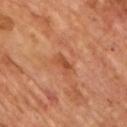Clinical impression:
Part of a total-body skin-imaging series; this lesion was reviewed on a skin check and was not flagged for biopsy.
Clinical summary:
A 15 mm close-up extracted from a 3D total-body photography capture. The patient is a male roughly 65 years of age. Approximately 3 mm at its widest. Captured under cross-polarized illumination. The total-body-photography lesion software estimated a lesion area of about 3 mm². The software also gave an average lesion color of about L≈49 a*≈26 b*≈37 (CIELAB), roughly 8 lightness units darker than nearby skin, and a normalized border contrast of about 6.5. The analysis additionally found border irregularity of about 3.5 on a 0–10 scale, a within-lesion color-variation index near 0/10, and radial color variation of about 0. And it measured a nevus-likeness score of about 0/100 and a lesion-detection confidence of about 100/100.